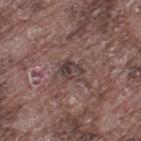- anatomic site · the leg
- imaging modality · ~15 mm crop, total-body skin-cancer survey
- illumination · white-light
- size · ≈2.5 mm
- subject · male, aged 73–77